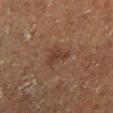Assessment:
Part of a total-body skin-imaging series; this lesion was reviewed on a skin check and was not flagged for biopsy.
Context:
A 15 mm close-up tile from a total-body photography series done for melanoma screening. Captured under cross-polarized illumination. The lesion is located on the left lower leg. About 3 mm across. A male patient aged 73–77.